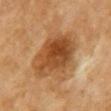• workup — imaged on a skin check; not biopsied
• acquisition — 15 mm crop, total-body photography
• tile lighting — cross-polarized
• site — the chest
• automated metrics — an area of roughly 24 mm² and two-axis asymmetry of about 0.15; a lesion color around L≈41 a*≈20 b*≈34 in CIELAB, about 11 CIELAB-L* units darker than the surrounding skin, and a normalized lesion–skin contrast near 9; border irregularity of about 2 on a 0–10 scale, a color-variation rating of about 6/10, and a peripheral color-asymmetry measure near 2; a nevus-likeness score of about 100/100 and a lesion-detection confidence of about 100/100
• lesion diameter — ≈7 mm
• patient — female, in their mid-50s